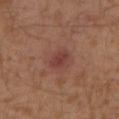| field | value |
|---|---|
| notes | catalogued during a skin exam; not biopsied |
| tile lighting | cross-polarized |
| patient | male, approximately 30 years of age |
| anatomic site | the left lower leg |
| automated lesion analysis | a lesion color around L≈38 a*≈24 b*≈22 in CIELAB and a lesion-to-skin contrast of about 7 (normalized; higher = more distinct); a within-lesion color-variation index near 2/10 and radial color variation of about 1; a classifier nevus-likeness of about 5/100 and a lesion-detection confidence of about 100/100 |
| image source | ~15 mm crop, total-body skin-cancer survey |